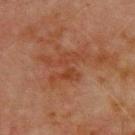biopsy_status: not biopsied; imaged during a skin examination
image:
  source: total-body photography crop
  field_of_view_mm: 15
patient:
  sex: male
  age_approx: 70
lesion_size:
  long_diameter_mm_approx: 4.0
site: chest
automated_metrics:
  area_mm2_approx: 7.0
  eccentricity: 0.75
  shape_asymmetry: 0.5
  nevus_likeness_0_100: 0